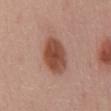No biopsy was performed on this lesion — it was imaged during a full skin examination and was not determined to be concerning. The tile uses white-light illumination. Automated image analysis of the tile measured roughly 14 lightness units darker than nearby skin and a normalized border contrast of about 10. It also reported a border-irregularity index near 1.5/10 and radial color variation of about 1.5. A close-up tile cropped from a whole-body skin photograph, about 15 mm across. The recorded lesion diameter is about 5.5 mm. A male subject aged 58 to 62. Located on the chest.